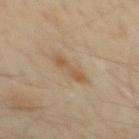workup — catalogued during a skin exam; not biopsied | automated metrics — an area of roughly 4.5 mm², an outline eccentricity of about 0.95 (0 = round, 1 = elongated), and two-axis asymmetry of about 0.35; about 8 CIELAB-L* units darker than the surrounding skin and a normalized border contrast of about 6.5; an automated nevus-likeness rating near 0 out of 100 and a lesion-detection confidence of about 100/100 | patient — male, roughly 55 years of age | site — the mid back | tile lighting — cross-polarized illumination | image — ~15 mm tile from a whole-body skin photo.